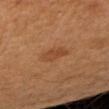{"biopsy_status": "not biopsied; imaged during a skin examination", "lesion_size": {"long_diameter_mm_approx": 3.0}, "site": "right upper arm", "patient": {"sex": "female", "age_approx": 40}, "lighting": "cross-polarized", "image": {"source": "total-body photography crop", "field_of_view_mm": 15}}